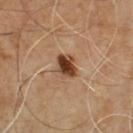Captured under cross-polarized illumination.
Cropped from a total-body skin-imaging series; the visible field is about 15 mm.
Located on the chest.
A male subject in their mid-40s.
About 3 mm across.
Automated image analysis of the tile measured a footprint of about 6 mm², a shape eccentricity near 0.65, and a shape-asymmetry score of about 0.3 (0 = symmetric). The software also gave border irregularity of about 2.5 on a 0–10 scale, a color-variation rating of about 5/10, and peripheral color asymmetry of about 1.5. The analysis additionally found a classifier nevus-likeness of about 95/100.